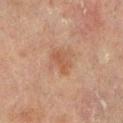Approximately 3 mm at its widest.
From the left lower leg.
The subject is a male approximately 70 years of age.
Automated image analysis of the tile measured an eccentricity of roughly 0.7 and two-axis asymmetry of about 0.35. The analysis additionally found an average lesion color of about L≈44 a*≈19 b*≈28 (CIELAB), a lesion–skin lightness drop of about 6, and a lesion-to-skin contrast of about 5.5 (normalized; higher = more distinct). The analysis additionally found a within-lesion color-variation index near 2/10 and radial color variation of about 0.5. The software also gave a detector confidence of about 100 out of 100 that the crop contains a lesion.
The tile uses cross-polarized illumination.
A 15 mm close-up tile from a total-body photography series done for melanoma screening.